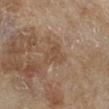  site: right lower leg
  image:
    source: total-body photography crop
    field_of_view_mm: 15
  patient:
    sex: female
    age_approx: 60